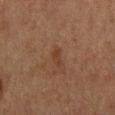workup: catalogued during a skin exam; not biopsied
lighting: cross-polarized
body site: the mid back
subject: male, in their mid- to late 70s
size: about 3 mm
imaging modality: ~15 mm crop, total-body skin-cancer survey